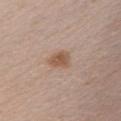Case summary:
* workup: imaged on a skin check; not biopsied
* automated lesion analysis: an area of roughly 5.5 mm², an outline eccentricity of about 0.7 (0 = round, 1 = elongated), and two-axis asymmetry of about 0.25; a nevus-likeness score of about 80/100 and a lesion-detection confidence of about 100/100
* patient: female, aged around 25
* acquisition: ~15 mm tile from a whole-body skin photo
* anatomic site: the upper back
* lighting: white-light illumination
* lesion diameter: ~3 mm (longest diameter)